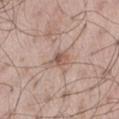Assessment:
The lesion was tiled from a total-body skin photograph and was not biopsied.
Acquisition and patient details:
Imaged with white-light lighting. A male subject in their mid- to late 50s. Located on the leg. A 15 mm close-up tile from a total-body photography series done for melanoma screening. About 3 mm across.